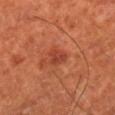workup: no biopsy performed (imaged during a skin exam); subject: male, about 70 years old; acquisition: total-body-photography crop, ~15 mm field of view; lighting: cross-polarized; lesion size: ≈3 mm; anatomic site: the leg.